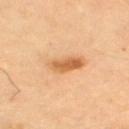<lesion>
<biopsy_status>not biopsied; imaged during a skin examination</biopsy_status>
<automated_metrics>
  <area_mm2_approx>12.0</area_mm2_approx>
  <eccentricity>0.8</eccentricity>
  <nevus_likeness_0_100>90</nevus_likeness_0_100>
  <lesion_detection_confidence_0_100>100</lesion_detection_confidence_0_100>
</automated_metrics>
<patient>
  <sex>female</sex>
  <age_approx>70</age_approx>
</patient>
<lesion_size>
  <long_diameter_mm_approx>5.0</long_diameter_mm_approx>
</lesion_size>
<image>
  <source>total-body photography crop</source>
  <field_of_view_mm>15</field_of_view_mm>
</image>
<lighting>cross-polarized</lighting>
<site>left thigh</site>
</lesion>